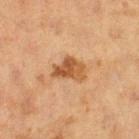Recorded during total-body skin imaging; not selected for excision or biopsy.
From the left thigh.
The tile uses cross-polarized illumination.
A female subject, aged 38–42.
About 3.5 mm across.
A roughly 15 mm field-of-view crop from a total-body skin photograph.